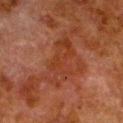Recorded during total-body skin imaging; not selected for excision or biopsy.
A male patient, aged 78 to 82.
The total-body-photography lesion software estimated an area of roughly 15 mm² and a symmetry-axis asymmetry near 0.3. The software also gave an average lesion color of about L≈30 a*≈23 b*≈28 (CIELAB), roughly 6 lightness units darker than nearby skin, and a normalized border contrast of about 6.5. It also reported border irregularity of about 5.5 on a 0–10 scale, a within-lesion color-variation index near 4/10, and a peripheral color-asymmetry measure near 1.5.
Captured under cross-polarized illumination.
From the left lower leg.
Cropped from a total-body skin-imaging series; the visible field is about 15 mm.